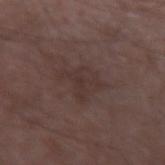notes: total-body-photography surveillance lesion; no biopsy | automated lesion analysis: a border-irregularity index near 6/10, internal color variation of about 2 on a 0–10 scale, and a peripheral color-asymmetry measure near 0.5 | lighting: white-light | imaging modality: ~15 mm tile from a whole-body skin photo | site: the right forearm | size: ~4 mm (longest diameter) | patient: male, roughly 75 years of age.